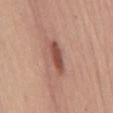{"biopsy_status": "not biopsied; imaged during a skin examination", "site": "front of the torso", "patient": {"sex": "male", "age_approx": 60}, "automated_metrics": {"area_mm2_approx": 7.5, "shape_asymmetry": 0.25, "border_irregularity_0_10": 3.0, "color_variation_0_10": 3.0, "peripheral_color_asymmetry": 1.0}, "image": {"source": "total-body photography crop", "field_of_view_mm": 15}, "lighting": "white-light"}